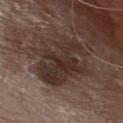Q: Is there a histopathology result?
A: catalogued during a skin exam; not biopsied
Q: How was this image acquired?
A: 15 mm crop, total-body photography
Q: Illumination type?
A: white-light illumination
Q: Who is the patient?
A: male, roughly 75 years of age
Q: Lesion location?
A: the chest